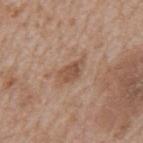This lesion was catalogued during total-body skin photography and was not selected for biopsy.
Imaged with white-light lighting.
Located on the mid back.
Approximately 3.5 mm at its widest.
A male subject aged around 65.
Automated image analysis of the tile measured a border-irregularity index near 2.5/10, a within-lesion color-variation index near 3/10, and radial color variation of about 1.
A 15 mm close-up extracted from a 3D total-body photography capture.